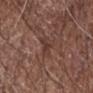notes=total-body-photography surveillance lesion; no biopsy | body site=the right forearm | image source=~15 mm tile from a whole-body skin photo | size=~2.5 mm (longest diameter) | TBP lesion metrics=an average lesion color of about L≈37 a*≈19 b*≈23 (CIELAB), a lesion–skin lightness drop of about 6, and a normalized lesion–skin contrast near 5.5 | illumination=white-light illumination | subject=male, aged 68–72.